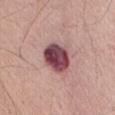Q: What is the lesion's diameter?
A: ≈4 mm
Q: Where on the body is the lesion?
A: the abdomen
Q: What are the patient's age and sex?
A: male, aged 63 to 67
Q: Illumination type?
A: white-light
Q: What did automated image analysis measure?
A: a shape-asymmetry score of about 0.1 (0 = symmetric); a border-irregularity index near 1.5/10, a within-lesion color-variation index near 8/10, and peripheral color asymmetry of about 2.5
Q: How was this image acquired?
A: ~15 mm crop, total-body skin-cancer survey